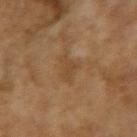Findings:
- biopsy status: total-body-photography surveillance lesion; no biopsy
- body site: the left upper arm
- lesion size: ~2.5 mm (longest diameter)
- illumination: cross-polarized
- acquisition: total-body-photography crop, ~15 mm field of view
- TBP lesion metrics: a border-irregularity index near 3.5/10; a classifier nevus-likeness of about 0/100 and a lesion-detection confidence of about 100/100
- subject: female, in their 60s